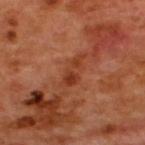{
  "biopsy_status": "not biopsied; imaged during a skin examination",
  "patient": {
    "sex": "male",
    "age_approx": 50
  },
  "image": {
    "source": "total-body photography crop",
    "field_of_view_mm": 15
  },
  "site": "upper back",
  "lighting": "cross-polarized"
}